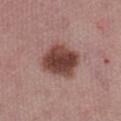Case summary:
– image source: ~15 mm crop, total-body skin-cancer survey
– site: the abdomen
– subject: female, aged 53 to 57
– tile lighting: white-light illumination
– size: ≈5 mm